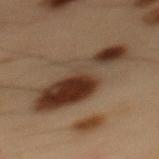The lesion was photographed on a routine skin check and not biopsied; there is no pathology result. The tile uses cross-polarized illumination. The recorded lesion diameter is about 10 mm. A male subject in their mid-50s. Cropped from a total-body skin-imaging series; the visible field is about 15 mm. Automated image analysis of the tile measured an average lesion color of about L≈29 a*≈13 b*≈22 (CIELAB). It also reported border irregularity of about 5.5 on a 0–10 scale, a color-variation rating of about 9.5/10, and a peripheral color-asymmetry measure near 4. On the mid back.